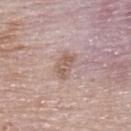Impression:
Imaged during a routine full-body skin examination; the lesion was not biopsied and no histopathology is available.
Background:
A lesion tile, about 15 mm wide, cut from a 3D total-body photograph. From the upper back. Measured at roughly 3.5 mm in maximum diameter. This is a white-light tile. The lesion-visualizer software estimated a lesion area of about 5.5 mm², an outline eccentricity of about 0.85 (0 = round, 1 = elongated), and a shape-asymmetry score of about 0.2 (0 = symmetric). The software also gave a lesion color around L≈58 a*≈17 b*≈25 in CIELAB and roughly 9 lightness units darker than nearby skin. And it measured a classifier nevus-likeness of about 0/100 and lesion-presence confidence of about 100/100. A male subject, in their mid-80s or older.